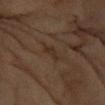Clinical impression: Imaged during a routine full-body skin examination; the lesion was not biopsied and no histopathology is available. Context: A female subject, aged approximately 80. A roughly 15 mm field-of-view crop from a total-body skin photograph. Located on the left forearm. The tile uses cross-polarized illumination.